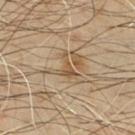No biopsy was performed on this lesion — it was imaged during a full skin examination and was not determined to be concerning. The total-body-photography lesion software estimated a mean CIELAB color near L≈50 a*≈14 b*≈32, about 9 CIELAB-L* units darker than the surrounding skin, and a normalized border contrast of about 7. The analysis additionally found a nevus-likeness score of about 0/100 and a detector confidence of about 50 out of 100 that the crop contains a lesion. A 15 mm close-up extracted from a 3D total-body photography capture. From the chest. Measured at roughly 3 mm in maximum diameter. The subject is a male approximately 55 years of age. The tile uses cross-polarized illumination.